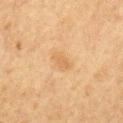TBP lesion metrics: a mean CIELAB color near L≈52 a*≈16 b*≈35, roughly 6 lightness units darker than nearby skin, and a lesion-to-skin contrast of about 5 (normalized; higher = more distinct)
patient: male, aged approximately 65
body site: the right upper arm
lesion size: about 2.5 mm
illumination: cross-polarized
image: ~15 mm tile from a whole-body skin photo